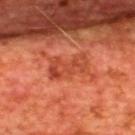Q: Was a biopsy performed?
A: catalogued during a skin exam; not biopsied
Q: What kind of image is this?
A: ~15 mm crop, total-body skin-cancer survey
Q: Lesion location?
A: the back
Q: What lighting was used for the tile?
A: cross-polarized
Q: Automated lesion metrics?
A: a lesion color around L≈42 a*≈32 b*≈35 in CIELAB and a normalized lesion–skin contrast near 5.5
Q: Who is the patient?
A: male, aged 63–67